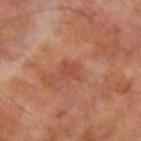The lesion was tiled from a total-body skin photograph and was not biopsied.
A 15 mm close-up extracted from a 3D total-body photography capture.
Automated image analysis of the tile measured a shape eccentricity near 0.75 and a shape-asymmetry score of about 0.3 (0 = symmetric). And it measured about 7 CIELAB-L* units darker than the surrounding skin and a lesion-to-skin contrast of about 5.5 (normalized; higher = more distinct). The analysis additionally found a border-irregularity rating of about 3/10, a within-lesion color-variation index near 1/10, and radial color variation of about 0.5. The software also gave a classifier nevus-likeness of about 0/100 and lesion-presence confidence of about 100/100.
The lesion is on the left upper arm.
This is a cross-polarized tile.
The subject is a male aged 68 to 72.